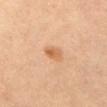This lesion was catalogued during total-body skin photography and was not selected for biopsy. A female subject, roughly 65 years of age. Captured under cross-polarized illumination. The lesion is on the front of the torso. A close-up tile cropped from a whole-body skin photograph, about 15 mm across. The lesion's longest dimension is about 2.5 mm.